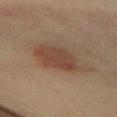Q: Lesion size?
A: about 7.5 mm
Q: What kind of image is this?
A: ~15 mm tile from a whole-body skin photo
Q: Illumination type?
A: cross-polarized
Q: Where on the body is the lesion?
A: the mid back
Q: Who is the patient?
A: female, aged 43 to 47
Q: What did automated image analysis measure?
A: an average lesion color of about L≈38 a*≈15 b*≈24 (CIELAB), roughly 8 lightness units darker than nearby skin, and a normalized border contrast of about 7.5; border irregularity of about 3 on a 0–10 scale, a within-lesion color-variation index near 3.5/10, and a peripheral color-asymmetry measure near 1; a classifier nevus-likeness of about 100/100 and a lesion-detection confidence of about 100/100